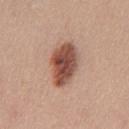Imaged during a routine full-body skin examination; the lesion was not biopsied and no histopathology is available.
A lesion tile, about 15 mm wide, cut from a 3D total-body photograph.
A male subject, approximately 30 years of age.
The lesion is located on the chest.